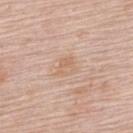The lesion was tiled from a total-body skin photograph and was not biopsied. The subject is a female approximately 65 years of age. This is a white-light tile. From the upper back. A 15 mm close-up extracted from a 3D total-body photography capture. The recorded lesion diameter is about 2.5 mm.